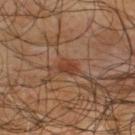A roughly 15 mm field-of-view crop from a total-body skin photograph.
Measured at roughly 2.5 mm in maximum diameter.
The lesion is on the upper back.
A male subject aged around 65.